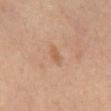patient = female, aged around 50
illumination = cross-polarized illumination
acquisition = ~15 mm crop, total-body skin-cancer survey
body site = the mid back
lesion diameter = about 2.5 mm